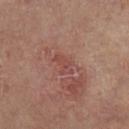Findings:
• biopsy status: no biopsy performed (imaged during a skin exam)
• site: the right thigh
• patient: male, aged 83 or older
• acquisition: ~15 mm tile from a whole-body skin photo